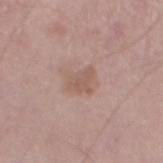<lesion>
  <biopsy_status>not biopsied; imaged during a skin examination</biopsy_status>
  <automated_metrics>
    <area_mm2_approx>5.0</area_mm2_approx>
    <eccentricity>0.6</eccentricity>
    <cielab_L>56</cielab_L>
    <cielab_a>18</cielab_a>
    <cielab_b>25</cielab_b>
    <vs_skin_darker_L>7.0</vs_skin_darker_L>
    <vs_skin_contrast_norm>5.5</vs_skin_contrast_norm>
    <border_irregularity_0_10>4.0</border_irregularity_0_10>
    <peripheral_color_asymmetry>0.5</peripheral_color_asymmetry>
  </automated_metrics>
  <lighting>white-light</lighting>
  <image>
    <source>total-body photography crop</source>
    <field_of_view_mm>15</field_of_view_mm>
  </image>
  <patient>
    <sex>male</sex>
    <age_approx>70</age_approx>
  </patient>
  <site>left thigh</site>
</lesion>